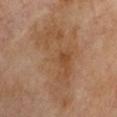This lesion was catalogued during total-body skin photography and was not selected for biopsy. A male subject, aged 68 to 72. A roughly 15 mm field-of-view crop from a total-body skin photograph. The lesion-visualizer software estimated a lesion area of about 38 mm² and a shape-asymmetry score of about 0.45 (0 = symmetric). The analysis additionally found a detector confidence of about 100 out of 100 that the crop contains a lesion. Approximately 11 mm at its widest. The lesion is located on the right upper arm.